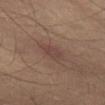Findings:
– biopsy status: imaged on a skin check; not biopsied
– lighting: cross-polarized
– diameter: ~3.5 mm (longest diameter)
– image: ~15 mm tile from a whole-body skin photo
– subject: male, aged 58–62
– automated lesion analysis: a lesion area of about 5 mm², an eccentricity of roughly 0.9, and a shape-asymmetry score of about 0.35 (0 = symmetric)
– body site: the leg